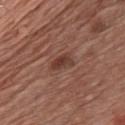Findings:
- biopsy status · no biopsy performed (imaged during a skin exam)
- lesion size · ~3 mm (longest diameter)
- illumination · white-light illumination
- subject · male, approximately 65 years of age
- body site · the front of the torso
- acquisition · 15 mm crop, total-body photography
- TBP lesion metrics · a lesion color around L≈38 a*≈22 b*≈25 in CIELAB, a lesion–skin lightness drop of about 9, and a lesion-to-skin contrast of about 8 (normalized; higher = more distinct); a classifier nevus-likeness of about 65/100 and lesion-presence confidence of about 100/100